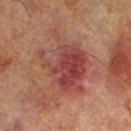The lesion was tiled from a total-body skin photograph and was not biopsied.
A male patient aged approximately 70.
About 7.5 mm across.
This is a cross-polarized tile.
A 15 mm close-up extracted from a 3D total-body photography capture.
On the left lower leg.